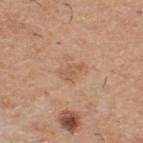Q: Was a biopsy performed?
A: catalogued during a skin exam; not biopsied
Q: Illumination type?
A: white-light illumination
Q: Automated lesion metrics?
A: a lesion color around L≈57 a*≈20 b*≈33 in CIELAB, about 7 CIELAB-L* units darker than the surrounding skin, and a lesion-to-skin contrast of about 5 (normalized; higher = more distinct); a classifier nevus-likeness of about 0/100
Q: Lesion size?
A: about 3 mm
Q: What are the patient's age and sex?
A: male, roughly 60 years of age
Q: How was this image acquired?
A: ~15 mm crop, total-body skin-cancer survey
Q: Lesion location?
A: the upper back